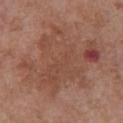{
  "biopsy_status": "not biopsied; imaged during a skin examination",
  "site": "chest",
  "lighting": "white-light",
  "automated_metrics": {
    "area_mm2_approx": 50.0,
    "eccentricity": 0.7,
    "shape_asymmetry": 0.35,
    "border_irregularity_0_10": 7.5,
    "color_variation_0_10": 6.0,
    "peripheral_color_asymmetry": 2.0
  },
  "patient": {
    "sex": "female",
    "age_approx": 75
  },
  "image": {
    "source": "total-body photography crop",
    "field_of_view_mm": 15
  },
  "lesion_size": {
    "long_diameter_mm_approx": 9.5
  }
}